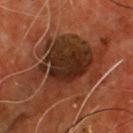The lesion was photographed on a routine skin check and not biopsied; there is no pathology result. Automated image analysis of the tile measured a lesion area of about 45 mm², an outline eccentricity of about 0.75 (0 = round, 1 = elongated), and a shape-asymmetry score of about 0.35 (0 = symmetric). It also reported a classifier nevus-likeness of about 0/100 and a lesion-detection confidence of about 95/100. Captured under cross-polarized illumination. The patient is a male aged around 55. On the chest. About 11.5 mm across. A 15 mm close-up extracted from a 3D total-body photography capture.